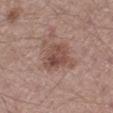Notes:
• notes — no biopsy performed (imaged during a skin exam)
• tile lighting — white-light illumination
• subject — male, aged around 40
• lesion size — about 5 mm
• body site — the left lower leg
• automated metrics — an area of roughly 14 mm², an outline eccentricity of about 0.65 (0 = round, 1 = elongated), and two-axis asymmetry of about 0.35; a mean CIELAB color near L≈49 a*≈19 b*≈24 and about 10 CIELAB-L* units darker than the surrounding skin; a within-lesion color-variation index near 4.5/10 and a peripheral color-asymmetry measure near 1.5
• imaging modality — 15 mm crop, total-body photography